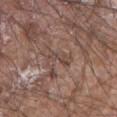No biopsy was performed on this lesion — it was imaged during a full skin examination and was not determined to be concerning.
About 3 mm across.
The total-body-photography lesion software estimated a lesion area of about 3.5 mm², an outline eccentricity of about 0.85 (0 = round, 1 = elongated), and a symmetry-axis asymmetry near 0.45. The analysis additionally found a lesion color around L≈45 a*≈16 b*≈22 in CIELAB, roughly 7 lightness units darker than nearby skin, and a normalized lesion–skin contrast near 5.5.
A region of skin cropped from a whole-body photographic capture, roughly 15 mm wide.
The subject is a male in their 80s.
Located on the right upper arm.